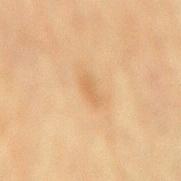Impression:
Captured during whole-body skin photography for melanoma surveillance; the lesion was not biopsied.
Acquisition and patient details:
Captured under cross-polarized illumination. This image is a 15 mm lesion crop taken from a total-body photograph. Approximately 2.5 mm at its widest. From the lower back. The subject is a female aged around 80.